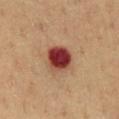<record>
<image>
  <source>total-body photography crop</source>
  <field_of_view_mm>15</field_of_view_mm>
</image>
<patient>
  <sex>male</sex>
  <age_approx>60</age_approx>
</patient>
<lighting>cross-polarized</lighting>
<site>chest</site>
</record>